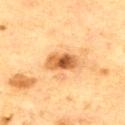Findings:
- acquisition — 15 mm crop, total-body photography
- tile lighting — cross-polarized
- body site — the chest
- patient — male, roughly 65 years of age
- lesion size — about 3.5 mm